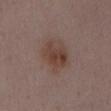Imaged during a routine full-body skin examination; the lesion was not biopsied and no histopathology is available. The tile uses white-light illumination. The patient is a female roughly 30 years of age. Measured at roughly 4.5 mm in maximum diameter. The lesion is located on the chest. Automated image analysis of the tile measured an eccentricity of roughly 0.5. It also reported a mean CIELAB color near L≈41 a*≈17 b*≈23, a lesion–skin lightness drop of about 8, and a normalized lesion–skin contrast near 7.5. A lesion tile, about 15 mm wide, cut from a 3D total-body photograph.